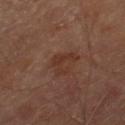Impression:
The lesion was tiled from a total-body skin photograph and was not biopsied.
Clinical summary:
Captured under cross-polarized illumination. A region of skin cropped from a whole-body photographic capture, roughly 15 mm wide. A male subject, aged approximately 70. From the right thigh.